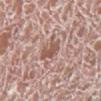Case summary:
• notes: total-body-photography surveillance lesion; no biopsy
• lighting: white-light illumination
• location: the right lower leg
• patient: male, aged around 75
• automated lesion analysis: an area of roughly 5.5 mm², a shape eccentricity near 0.2, and a symmetry-axis asymmetry near 0.25; border irregularity of about 3.5 on a 0–10 scale and a color-variation rating of about 2.5/10; a nevus-likeness score of about 0/100 and a lesion-detection confidence of about 90/100
• imaging modality: ~15 mm crop, total-body skin-cancer survey